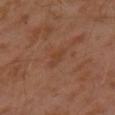No biopsy was performed on this lesion — it was imaged during a full skin examination and was not determined to be concerning. The patient is a male about 30 years old. Located on the left upper arm. About 3 mm across. The tile uses cross-polarized illumination. A close-up tile cropped from a whole-body skin photograph, about 15 mm across. Automated tile analysis of the lesion measured an area of roughly 3 mm², a shape eccentricity near 0.9, and a symmetry-axis asymmetry near 0.35. The software also gave an automated nevus-likeness rating near 0 out of 100 and a lesion-detection confidence of about 100/100.